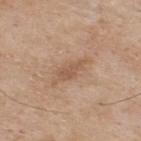The lesion was photographed on a routine skin check and not biopsied; there is no pathology result. A male subject, aged approximately 75. Cropped from a total-body skin-imaging series; the visible field is about 15 mm. The lesion is on the upper back. Imaged with white-light lighting. Longest diameter approximately 3.5 mm.